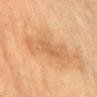Impression:
Captured during whole-body skin photography for melanoma surveillance; the lesion was not biopsied.
Context:
The total-body-photography lesion software estimated a lesion color around L≈54 a*≈19 b*≈36 in CIELAB, a lesion–skin lightness drop of about 7, and a lesion-to-skin contrast of about 5 (normalized; higher = more distinct). The recorded lesion diameter is about 7 mm. The tile uses cross-polarized illumination. Located on the front of the torso. A male patient about 85 years old or older. Cropped from a whole-body photographic skin survey; the tile spans about 15 mm.